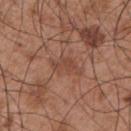No biopsy was performed on this lesion — it was imaged during a full skin examination and was not determined to be concerning.
A roughly 15 mm field-of-view crop from a total-body skin photograph.
A male patient in their mid- to late 50s.
On the chest.